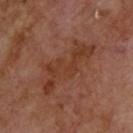The lesion was tiled from a total-body skin photograph and was not biopsied.
A male patient aged approximately 70.
The total-body-photography lesion software estimated a footprint of about 16 mm², an eccentricity of roughly 0.95, and two-axis asymmetry of about 0.5. The analysis additionally found border irregularity of about 8.5 on a 0–10 scale, internal color variation of about 3 on a 0–10 scale, and peripheral color asymmetry of about 1. The analysis additionally found a nevus-likeness score of about 0/100.
Located on the upper back.
The tile uses cross-polarized illumination.
A 15 mm crop from a total-body photograph taken for skin-cancer surveillance.
Approximately 8 mm at its widest.